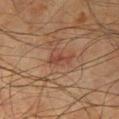Background: On the arm. The subject is a male roughly 75 years of age. The recorded lesion diameter is about 2.5 mm. A lesion tile, about 15 mm wide, cut from a 3D total-body photograph. Imaged with cross-polarized lighting.